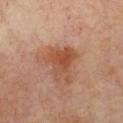Part of a total-body skin-imaging series; this lesion was reviewed on a skin check and was not flagged for biopsy. This is a cross-polarized tile. Cropped from a whole-body photographic skin survey; the tile spans about 15 mm. Measured at roughly 4 mm in maximum diameter. A male subject aged approximately 70. The lesion is located on the chest.